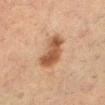{"biopsy_status": "not biopsied; imaged during a skin examination", "lighting": "cross-polarized", "patient": {"sex": "female", "age_approx": 55}, "image": {"source": "total-body photography crop", "field_of_view_mm": 15}, "site": "left lower leg", "lesion_size": {"long_diameter_mm_approx": 5.0}}